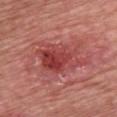Case summary:
• body site · the chest
• subject · male, aged approximately 60
• imaging modality · 15 mm crop, total-body photography